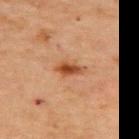| key | value |
|---|---|
| biopsy status | catalogued during a skin exam; not biopsied |
| anatomic site | the upper back |
| acquisition | total-body-photography crop, ~15 mm field of view |
| automated lesion analysis | a lesion area of about 4 mm², an outline eccentricity of about 0.85 (0 = round, 1 = elongated), and a symmetry-axis asymmetry near 0.2 |
| subject | female, aged 68 to 72 |
| diameter | ~3 mm (longest diameter) |
| tile lighting | cross-polarized |